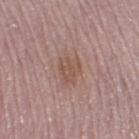biopsy status: no biopsy performed (imaged during a skin exam) | lesion diameter: ≈3 mm | site: the right thigh | image source: 15 mm crop, total-body photography | lighting: white-light | subject: male, approximately 65 years of age.